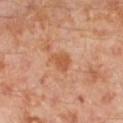follow-up: no biopsy performed (imaged during a skin exam) | lesion diameter: ~2.5 mm (longest diameter) | body site: the left lower leg | subject: male, aged around 30 | acquisition: 15 mm crop, total-body photography | image-analysis metrics: radial color variation of about 0.5.